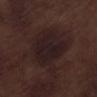follow-up: catalogued during a skin exam; not biopsied | body site: the left lower leg | illumination: white-light | image: ~15 mm tile from a whole-body skin photo | patient: male, aged around 70.